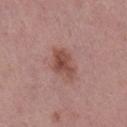Recorded during total-body skin imaging; not selected for excision or biopsy. Captured under white-light illumination. The patient is a female about 40 years old. A 15 mm close-up extracted from a 3D total-body photography capture. On the right lower leg.